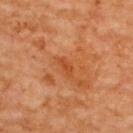| field | value |
|---|---|
| biopsy status | no biopsy performed (imaged during a skin exam) |
| image | ~15 mm crop, total-body skin-cancer survey |
| patient | female, in their mid-60s |
| lighting | cross-polarized |
| site | the upper back |